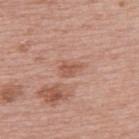{"biopsy_status": "not biopsied; imaged during a skin examination", "lighting": "white-light", "image": {"source": "total-body photography crop", "field_of_view_mm": 15}, "lesion_size": {"long_diameter_mm_approx": 2.5}, "patient": {"sex": "female", "age_approx": 60}, "site": "upper back"}